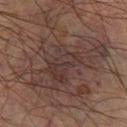No biopsy was performed on this lesion — it was imaged during a full skin examination and was not determined to be concerning.
Cropped from a total-body skin-imaging series; the visible field is about 15 mm.
Located on the right lower leg.
The subject is a male approximately 70 years of age.